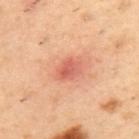Q: How was this image acquired?
A: 15 mm crop, total-body photography
Q: What are the patient's age and sex?
A: male, aged around 55
Q: Where on the body is the lesion?
A: the upper back
Q: Lesion size?
A: ≈3 mm
Q: How was the tile lit?
A: cross-polarized